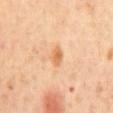<record>
<biopsy_status>not biopsied; imaged during a skin examination</biopsy_status>
<patient>
  <sex>female</sex>
  <age_approx>65</age_approx>
</patient>
<lighting>cross-polarized</lighting>
<site>mid back</site>
<image>
  <source>total-body photography crop</source>
  <field_of_view_mm>15</field_of_view_mm>
</image>
<lesion_size>
  <long_diameter_mm_approx>3.0</long_diameter_mm_approx>
</lesion_size>
</record>